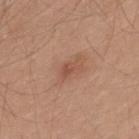{"biopsy_status": "not biopsied; imaged during a skin examination", "image": {"source": "total-body photography crop", "field_of_view_mm": 15}, "automated_metrics": {"area_mm2_approx": 3.0, "eccentricity": 0.85, "shape_asymmetry": 0.45, "cielab_L": 51, "cielab_a": 23, "cielab_b": 30, "vs_skin_darker_L": 8.0, "vs_skin_contrast_norm": 5.5, "color_variation_0_10": 1.0, "nevus_likeness_0_100": 50, "lesion_detection_confidence_0_100": 100}, "patient": {"sex": "male", "age_approx": 30}, "lighting": "white-light", "site": "upper back"}